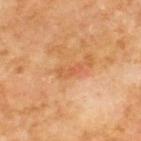follow-up: catalogued during a skin exam; not biopsied | tile lighting: cross-polarized illumination | image: 15 mm crop, total-body photography | site: the upper back | subject: male, roughly 70 years of age | TBP lesion metrics: a border-irregularity index near 4.5/10 and a within-lesion color-variation index near 0/10; lesion-presence confidence of about 100/100.